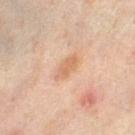Case summary:
* follow-up: total-body-photography surveillance lesion; no biopsy
* diameter: about 3 mm
* image-analysis metrics: a lesion color around L≈63 a*≈21 b*≈34 in CIELAB, a lesion–skin lightness drop of about 8, and a normalized border contrast of about 6; a nevus-likeness score of about 35/100 and a detector confidence of about 100 out of 100 that the crop contains a lesion
* location: the right thigh
* subject: female, approximately 50 years of age
* acquisition: ~15 mm tile from a whole-body skin photo